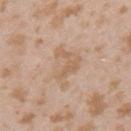No biopsy was performed on this lesion — it was imaged during a full skin examination and was not determined to be concerning.
The lesion is on the left upper arm.
A lesion tile, about 15 mm wide, cut from a 3D total-body photograph.
A female subject about 25 years old.
Measured at roughly 5 mm in maximum diameter.